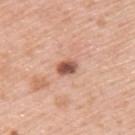Findings:
• notes — catalogued during a skin exam; not biopsied
• tile lighting — white-light illumination
• acquisition — ~15 mm crop, total-body skin-cancer survey
• subject — male, approximately 60 years of age
• lesion diameter — ~2.5 mm (longest diameter)
• site — the back
• image-analysis metrics — roughly 16 lightness units darker than nearby skin and a normalized border contrast of about 10; a nevus-likeness score of about 90/100 and a detector confidence of about 100 out of 100 that the crop contains a lesion